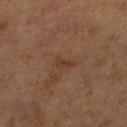Q: Is there a histopathology result?
A: no biopsy performed (imaged during a skin exam)
Q: Where on the body is the lesion?
A: the right lower leg
Q: What is the lesion's diameter?
A: ~2.5 mm (longest diameter)
Q: How was this image acquired?
A: 15 mm crop, total-body photography
Q: Who is the patient?
A: male, in their 70s
Q: Illumination type?
A: cross-polarized